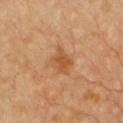Impression:
Imaged during a routine full-body skin examination; the lesion was not biopsied and no histopathology is available.
Background:
On the chest. Automated image analysis of the tile measured an area of roughly 6 mm². The software also gave a color-variation rating of about 2.5/10 and radial color variation of about 0.5. The analysis additionally found lesion-presence confidence of about 100/100. About 3.5 mm across. A roughly 15 mm field-of-view crop from a total-body skin photograph. Captured under cross-polarized illumination.